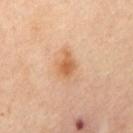Impression: The lesion was tiled from a total-body skin photograph and was not biopsied.